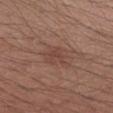biopsy status — catalogued during a skin exam; not biopsied
acquisition — 15 mm crop, total-body photography
automated metrics — an automated nevus-likeness rating near 0 out of 100 and lesion-presence confidence of about 100/100
patient — male, approximately 30 years of age
body site — the right forearm
tile lighting — white-light illumination